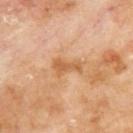Q: Is there a histopathology result?
A: no biopsy performed (imaged during a skin exam)
Q: Who is the patient?
A: male, approximately 70 years of age
Q: How large is the lesion?
A: about 3.5 mm
Q: Lesion location?
A: the upper back
Q: How was this image acquired?
A: ~15 mm crop, total-body skin-cancer survey
Q: How was the tile lit?
A: cross-polarized